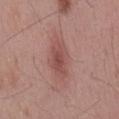Part of a total-body skin-imaging series; this lesion was reviewed on a skin check and was not flagged for biopsy.
A region of skin cropped from a whole-body photographic capture, roughly 15 mm wide.
From the mid back.
Automated tile analysis of the lesion measured a mean CIELAB color near L≈50 a*≈24 b*≈23 and a normalized lesion–skin contrast near 6.5. It also reported an automated nevus-likeness rating near 70 out of 100 and lesion-presence confidence of about 100/100.
The tile uses white-light illumination.
The lesion's longest dimension is about 4.5 mm.
A male patient aged 53–57.